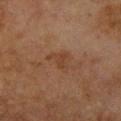The lesion was tiled from a total-body skin photograph and was not biopsied.
The lesion is located on the right forearm.
The tile uses cross-polarized illumination.
Approximately 3 mm at its widest.
Automated tile analysis of the lesion measured an outline eccentricity of about 0.65 (0 = round, 1 = elongated). And it measured border irregularity of about 5.5 on a 0–10 scale, internal color variation of about 1 on a 0–10 scale, and a peripheral color-asymmetry measure near 0.5. The analysis additionally found a classifier nevus-likeness of about 0/100 and a detector confidence of about 100 out of 100 that the crop contains a lesion.
A roughly 15 mm field-of-view crop from a total-body skin photograph.
A female patient roughly 80 years of age.